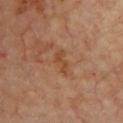Clinical impression: Part of a total-body skin-imaging series; this lesion was reviewed on a skin check and was not flagged for biopsy. Context: Located on the front of the torso. The tile uses cross-polarized illumination. Measured at roughly 3.5 mm in maximum diameter. A subject in their mid- to late 60s. Cropped from a total-body skin-imaging series; the visible field is about 15 mm.